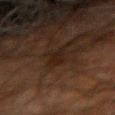Notes:
• biopsy status: total-body-photography surveillance lesion; no biopsy
• diameter: ≈3 mm
• image-analysis metrics: an outline eccentricity of about 0.8 (0 = round, 1 = elongated) and two-axis asymmetry of about 0.3; a classifier nevus-likeness of about 0/100 and a detector confidence of about 90 out of 100 that the crop contains a lesion
• acquisition: 15 mm crop, total-body photography
• lighting: cross-polarized
• patient: male, approximately 80 years of age
• location: the right forearm